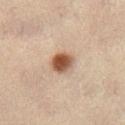<lesion>
<lesion_size>
  <long_diameter_mm_approx>3.0</long_diameter_mm_approx>
</lesion_size>
<image>
  <source>total-body photography crop</source>
  <field_of_view_mm>15</field_of_view_mm>
</image>
<patient>
  <sex>female</sex>
  <age_approx>60</age_approx>
</patient>
<site>abdomen</site>
</lesion>